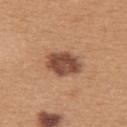Assessment:
Recorded during total-body skin imaging; not selected for excision or biopsy.
Clinical summary:
The patient is a female aged around 30. Located on the upper back. A region of skin cropped from a whole-body photographic capture, roughly 15 mm wide.